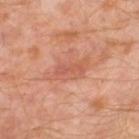No biopsy was performed on this lesion — it was imaged during a full skin examination and was not determined to be concerning.
This image is a 15 mm lesion crop taken from a total-body photograph.
The patient is a male aged approximately 30.
Located on the left thigh.